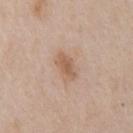notes: imaged on a skin check; not biopsied
image-analysis metrics: a within-lesion color-variation index near 2.5/10 and radial color variation of about 1; a detector confidence of about 100 out of 100 that the crop contains a lesion
tile lighting: white-light
anatomic site: the chest
subject: male, aged around 60
image source: ~15 mm tile from a whole-body skin photo